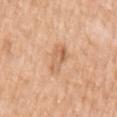Captured during whole-body skin photography for melanoma surveillance; the lesion was not biopsied. Automated image analysis of the tile measured a border-irregularity rating of about 3.5/10 and internal color variation of about 6 on a 0–10 scale. This image is a 15 mm lesion crop taken from a total-body photograph. The lesion is on the left upper arm. Longest diameter approximately 4.5 mm. A female subject in their mid-50s.